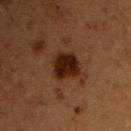A male patient, aged around 50. From the chest. Captured under cross-polarized illumination. The recorded lesion diameter is about 4 mm. A roughly 15 mm field-of-view crop from a total-body skin photograph.